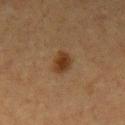Assessment: Imaged during a routine full-body skin examination; the lesion was not biopsied and no histopathology is available. Image and clinical context: A 15 mm close-up extracted from a 3D total-body photography capture. Located on the arm. Automated tile analysis of the lesion measured an area of roughly 5.5 mm² and an outline eccentricity of about 0.75 (0 = round, 1 = elongated). And it measured an average lesion color of about L≈30 a*≈16 b*≈28 (CIELAB) and about 8 CIELAB-L* units darker than the surrounding skin. The tile uses cross-polarized illumination. The lesion's longest dimension is about 3.5 mm. The patient is a female roughly 55 years of age.